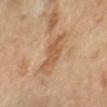The lesion was photographed on a routine skin check and not biopsied; there is no pathology result.
The lesion is located on the left forearm.
Automated image analysis of the tile measured a lesion area of about 9 mm², a shape eccentricity near 0.9, and two-axis asymmetry of about 0.35. And it measured a nevus-likeness score of about 0/100.
A region of skin cropped from a whole-body photographic capture, roughly 15 mm wide.
A female subject aged around 60.